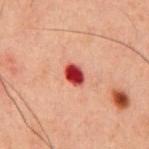Captured during whole-body skin photography for melanoma surveillance; the lesion was not biopsied.
The lesion-visualizer software estimated an area of roughly 5 mm², an outline eccentricity of about 0.7 (0 = round, 1 = elongated), and two-axis asymmetry of about 0.15. The software also gave a nevus-likeness score of about 0/100.
Approximately 2.5 mm at its widest.
On the front of the torso.
A male subject roughly 60 years of age.
A roughly 15 mm field-of-view crop from a total-body skin photograph.